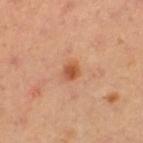Measured at roughly 2 mm in maximum diameter. Automated image analysis of the tile measured an eccentricity of roughly 0.55. The software also gave a border-irregularity rating of about 1.5/10 and a color-variation rating of about 4/10. Cropped from a total-body skin-imaging series; the visible field is about 15 mm. Captured under cross-polarized illumination. The lesion is located on the left thigh. The subject is a female in their mid- to late 30s.